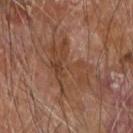Impression: Imaged during a routine full-body skin examination; the lesion was not biopsied and no histopathology is available. Image and clinical context: Captured under cross-polarized illumination. A roughly 15 mm field-of-view crop from a total-body skin photograph. The lesion is on the right forearm. Approximately 6 mm at its widest. A male subject aged 68–72. Automated image analysis of the tile measured a color-variation rating of about 4.5/10 and radial color variation of about 1.5. The analysis additionally found a lesion-detection confidence of about 80/100.